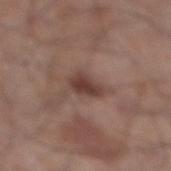This lesion was catalogued during total-body skin photography and was not selected for biopsy.